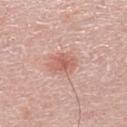Captured during whole-body skin photography for melanoma surveillance; the lesion was not biopsied. This is a white-light tile. A male patient, aged around 70. On the left lower leg. Automated tile analysis of the lesion measured an outline eccentricity of about 0.6 (0 = round, 1 = elongated) and a shape-asymmetry score of about 0.2 (0 = symmetric). It also reported a classifier nevus-likeness of about 90/100 and a lesion-detection confidence of about 100/100. A 15 mm close-up extracted from a 3D total-body photography capture.